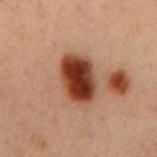Clinical impression:
Recorded during total-body skin imaging; not selected for excision or biopsy.
Image and clinical context:
About 5 mm across. A region of skin cropped from a whole-body photographic capture, roughly 15 mm wide. On the mid back. The patient is a male approximately 60 years of age. Imaged with cross-polarized lighting.